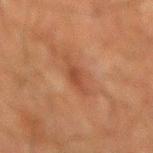biopsy status: imaged on a skin check; not biopsied | acquisition: ~15 mm tile from a whole-body skin photo | illumination: cross-polarized illumination | location: the mid back | subject: male, in their 60s | diameter: ~4.5 mm (longest diameter) | image-analysis metrics: a lesion area of about 4.5 mm², a shape eccentricity near 0.95, and a symmetry-axis asymmetry near 0.3; an average lesion color of about L≈36 a*≈20 b*≈28 (CIELAB), roughly 7 lightness units darker than nearby skin, and a normalized lesion–skin contrast near 6; a border-irregularity rating of about 4.5/10, internal color variation of about 1.5 on a 0–10 scale, and a peripheral color-asymmetry measure near 0.5.